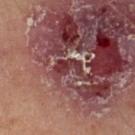Captured during whole-body skin photography for melanoma surveillance; the lesion was not biopsied.
Measured at roughly 3.5 mm in maximum diameter.
The subject is a male aged 68 to 72.
A 15 mm crop from a total-body photograph taken for skin-cancer surveillance.
The lesion is on the left lower leg.
Imaged with cross-polarized lighting.
Automated image analysis of the tile measured a footprint of about 5.5 mm² and a shape-asymmetry score of about 0.45 (0 = symmetric). The software also gave a lesion color around L≈36 a*≈27 b*≈19 in CIELAB, roughly 12 lightness units darker than nearby skin, and a lesion-to-skin contrast of about 9.5 (normalized; higher = more distinct). And it measured a color-variation rating of about 1.5/10 and radial color variation of about 0.5. It also reported a lesion-detection confidence of about 0/100.